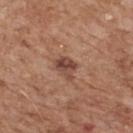Part of a total-body skin-imaging series; this lesion was reviewed on a skin check and was not flagged for biopsy.
A close-up tile cropped from a whole-body skin photograph, about 15 mm across.
Longest diameter approximately 3 mm.
The tile uses white-light illumination.
From the upper back.
The subject is a male in their mid- to late 60s.